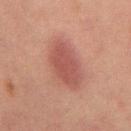Q: Lesion location?
A: the abdomen
Q: Who is the patient?
A: male, about 65 years old
Q: What is the imaging modality?
A: 15 mm crop, total-body photography
Q: What is the lesion's diameter?
A: about 5.5 mm
Q: How was the tile lit?
A: cross-polarized illumination